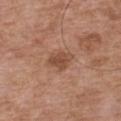workup: imaged on a skin check; not biopsied | anatomic site: the chest | image source: 15 mm crop, total-body photography | subject: male, aged 73 to 77 | automated lesion analysis: a mean CIELAB color near L≈48 a*≈22 b*≈31, a lesion–skin lightness drop of about 9, and a normalized lesion–skin contrast near 7; a border-irregularity rating of about 3/10, a within-lesion color-variation index near 2.5/10, and a peripheral color-asymmetry measure near 1; an automated nevus-likeness rating near 5 out of 100 and a detector confidence of about 100 out of 100 that the crop contains a lesion | illumination: white-light illumination | lesion diameter: ~3.5 mm (longest diameter).